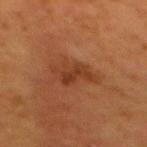No biopsy was performed on this lesion — it was imaged during a full skin examination and was not determined to be concerning.
Captured under cross-polarized illumination.
Located on the back.
Approximately 4.5 mm at its widest.
A female subject, aged 48–52.
The lesion-visualizer software estimated an area of roughly 7.5 mm², an outline eccentricity of about 0.75 (0 = round, 1 = elongated), and a symmetry-axis asymmetry near 0.4. The software also gave a classifier nevus-likeness of about 0/100 and a detector confidence of about 100 out of 100 that the crop contains a lesion.
This image is a 15 mm lesion crop taken from a total-body photograph.